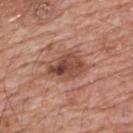tile lighting: white-light illumination
subject: male, aged around 80
location: the back
size: ~5 mm (longest diameter)
automated lesion analysis: a lesion area of about 13 mm² and a shape eccentricity near 0.7; a color-variation rating of about 8/10; a nevus-likeness score of about 40/100
imaging modality: ~15 mm crop, total-body skin-cancer survey